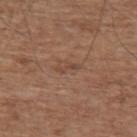Impression:
The lesion was photographed on a routine skin check and not biopsied; there is no pathology result.
Image and clinical context:
A male patient, roughly 75 years of age. Cropped from a total-body skin-imaging series; the visible field is about 15 mm. The recorded lesion diameter is about 2.5 mm. Imaged with white-light lighting. From the upper back.